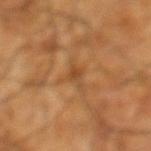The lesion was tiled from a total-body skin photograph and was not biopsied. The lesion is located on the left lower leg. An algorithmic analysis of the crop reported a footprint of about 3 mm² and a shape-asymmetry score of about 0.4 (0 = symmetric). The analysis additionally found a mean CIELAB color near L≈46 a*≈21 b*≈38, roughly 7 lightness units darker than nearby skin, and a lesion-to-skin contrast of about 6 (normalized; higher = more distinct). A male subject, approximately 60 years of age. The lesion's longest dimension is about 2.5 mm. A lesion tile, about 15 mm wide, cut from a 3D total-body photograph.